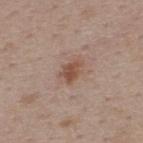<lesion>
<biopsy_status>not biopsied; imaged during a skin examination</biopsy_status>
<site>back</site>
<patient>
  <sex>female</sex>
  <age_approx>45</age_approx>
</patient>
<image>
  <source>total-body photography crop</source>
  <field_of_view_mm>15</field_of_view_mm>
</image>
<lighting>white-light</lighting>
<lesion_size>
  <long_diameter_mm_approx>3.0</long_diameter_mm_approx>
</lesion_size>
</lesion>